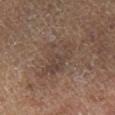Assessment:
The lesion was tiled from a total-body skin photograph and was not biopsied.
Acquisition and patient details:
Approximately 7 mm at its widest. A male subject approximately 65 years of age. A lesion tile, about 15 mm wide, cut from a 3D total-body photograph. The lesion is on the right lower leg. This is a cross-polarized tile.